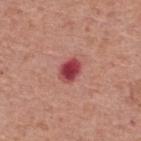This lesion was catalogued during total-body skin photography and was not selected for biopsy. The lesion's longest dimension is about 3 mm. The lesion is on the upper back. A 15 mm close-up extracted from a 3D total-body photography capture. This is a white-light tile. An algorithmic analysis of the crop reported a lesion area of about 5.5 mm², a shape eccentricity near 0.7, and two-axis asymmetry of about 0.15. The analysis additionally found a mean CIELAB color near L≈45 a*≈35 b*≈22 and roughly 16 lightness units darker than nearby skin. The analysis additionally found an automated nevus-likeness rating near 0 out of 100. A male subject, about 70 years old.